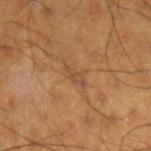Automated image analysis of the tile measured a lesion area of about 3 mm², an eccentricity of roughly 0.9, and two-axis asymmetry of about 0.35. It also reported a lesion color around L≈36 a*≈16 b*≈27 in CIELAB, roughly 5 lightness units darker than nearby skin, and a normalized border contrast of about 5. The analysis additionally found internal color variation of about 0 on a 0–10 scale. A male patient approximately 60 years of age. The tile uses cross-polarized illumination. Cropped from a whole-body photographic skin survey; the tile spans about 15 mm. Located on the arm. The lesion's longest dimension is about 3 mm.